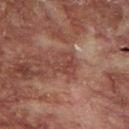Part of a total-body skin-imaging series; this lesion was reviewed on a skin check and was not flagged for biopsy. From the chest. A roughly 15 mm field-of-view crop from a total-body skin photograph. Automated tile analysis of the lesion measured an automated nevus-likeness rating near 0 out of 100 and lesion-presence confidence of about 65/100. This is a cross-polarized tile. A male patient, roughly 55 years of age. Longest diameter approximately 3.5 mm.